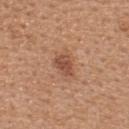acquisition: 15 mm crop, total-body photography
patient: female, in their mid-40s
automated metrics: border irregularity of about 3 on a 0–10 scale and a color-variation rating of about 2.5/10
site: the upper back
tile lighting: white-light